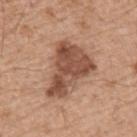<lesion>
<biopsy_status>not biopsied; imaged during a skin examination</biopsy_status>
<site>upper back</site>
<lesion_size>
  <long_diameter_mm_approx>6.5</long_diameter_mm_approx>
</lesion_size>
<image>
  <source>total-body photography crop</source>
  <field_of_view_mm>15</field_of_view_mm>
</image>
<patient>
  <sex>male</sex>
  <age_approx>55</age_approx>
</patient>
</lesion>